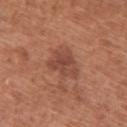Case summary:
• notes · catalogued during a skin exam; not biopsied
• anatomic site · the back
• subject · female, about 65 years old
• diameter · about 4 mm
• acquisition · ~15 mm crop, total-body skin-cancer survey
• tile lighting · white-light illumination